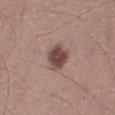Cropped from a whole-body photographic skin survey; the tile spans about 15 mm. Automated tile analysis of the lesion measured a footprint of about 7.5 mm², an outline eccentricity of about 0.55 (0 = round, 1 = elongated), and two-axis asymmetry of about 0.2. The software also gave a mean CIELAB color near L≈46 a*≈18 b*≈22, roughly 14 lightness units darker than nearby skin, and a normalized lesion–skin contrast near 10.5. The analysis additionally found a border-irregularity index near 1.5/10, internal color variation of about 3.5 on a 0–10 scale, and radial color variation of about 1. The lesion's longest dimension is about 3 mm. This is a white-light tile. From the left lower leg. A male patient aged approximately 45.